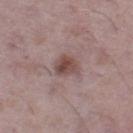Q: Was a biopsy performed?
A: catalogued during a skin exam; not biopsied
Q: Lesion size?
A: about 3.5 mm
Q: How was this image acquired?
A: 15 mm crop, total-body photography
Q: Who is the patient?
A: male, in their mid- to late 50s
Q: What is the anatomic site?
A: the left thigh